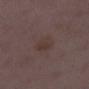workup = no biopsy performed (imaged during a skin exam) | acquisition = ~15 mm crop, total-body skin-cancer survey | body site = the left lower leg | patient = female, in their 30s.